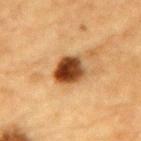Imaged during a routine full-body skin examination; the lesion was not biopsied and no histopathology is available. The lesion is on the mid back. This is a cross-polarized tile. Approximately 4 mm at its widest. A male patient, in their mid-80s or older. This image is a 15 mm lesion crop taken from a total-body photograph.